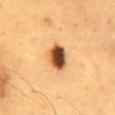Q: Was this lesion biopsied?
A: no biopsy performed (imaged during a skin exam)
Q: Automated lesion metrics?
A: a lesion area of about 7.5 mm², a shape eccentricity near 0.7, and a symmetry-axis asymmetry near 0.15; an automated nevus-likeness rating near 100 out of 100 and a lesion-detection confidence of about 100/100
Q: How was this image acquired?
A: total-body-photography crop, ~15 mm field of view
Q: What is the lesion's diameter?
A: ~4 mm (longest diameter)
Q: Patient demographics?
A: male, aged 58–62
Q: What is the anatomic site?
A: the back